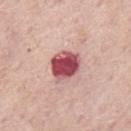| field | value |
|---|---|
| notes | no biopsy performed (imaged during a skin exam) |
| body site | the mid back |
| automated metrics | about 21 CIELAB-L* units darker than the surrounding skin and a normalized border contrast of about 13.5; border irregularity of about 1.5 on a 0–10 scale and peripheral color asymmetry of about 1.5; a nevus-likeness score of about 0/100 and lesion-presence confidence of about 100/100 |
| acquisition | ~15 mm crop, total-body skin-cancer survey |
| patient | male, in their mid- to late 70s |
| illumination | white-light |